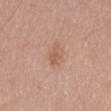Context: Measured at roughly 3 mm in maximum diameter. Captured under white-light illumination. The patient is a male roughly 75 years of age. An algorithmic analysis of the crop reported an area of roughly 4 mm², an eccentricity of roughly 0.85, and a symmetry-axis asymmetry near 0.3. The software also gave a border-irregularity rating of about 3/10, a color-variation rating of about 1.5/10, and peripheral color asymmetry of about 0.5. Cropped from a total-body skin-imaging series; the visible field is about 15 mm. From the right thigh.